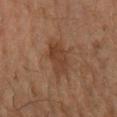biopsy status = no biopsy performed (imaged during a skin exam)
image = total-body-photography crop, ~15 mm field of view
site = the right forearm
subject = male, in their mid-40s
TBP lesion metrics = a within-lesion color-variation index near 3/10; a classifier nevus-likeness of about 5/100 and a detector confidence of about 100 out of 100 that the crop contains a lesion
lesion size = ≈4.5 mm